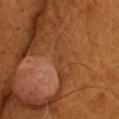No biopsy was performed on this lesion — it was imaged during a full skin examination and was not determined to be concerning.
The lesion's longest dimension is about 1.5 mm.
Captured under cross-polarized illumination.
The patient is a male in their mid-60s.
An algorithmic analysis of the crop reported a lesion area of about 1.5 mm², a shape eccentricity near 0.55, and a shape-asymmetry score of about 0.2 (0 = symmetric). It also reported a lesion color around L≈41 a*≈24 b*≈37 in CIELAB and a lesion-to-skin contrast of about 4 (normalized; higher = more distinct). The analysis additionally found border irregularity of about 1.5 on a 0–10 scale.
A close-up tile cropped from a whole-body skin photograph, about 15 mm across.
On the head or neck.